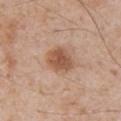Notes:
– follow-up · no biopsy performed (imaged during a skin exam)
– illumination · white-light
– image · total-body-photography crop, ~15 mm field of view
– subject · male, about 60 years old
– location · the chest